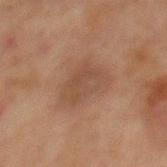Captured during whole-body skin photography for melanoma surveillance; the lesion was not biopsied. Captured under cross-polarized illumination. Cropped from a whole-body photographic skin survey; the tile spans about 15 mm. Located on the front of the torso. A male subject about 65 years old.